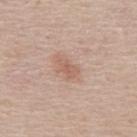{"biopsy_status": "not biopsied; imaged during a skin examination", "lighting": "white-light", "site": "mid back", "automated_metrics": {"cielab_L": 59, "cielab_a": 21, "cielab_b": 28, "vs_skin_darker_L": 8.0, "vs_skin_contrast_norm": 5.5}, "image": {"source": "total-body photography crop", "field_of_view_mm": 15}, "patient": {"sex": "male", "age_approx": 45}}